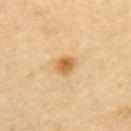follow-up = no biopsy performed (imaged during a skin exam)
patient = female, approximately 45 years of age
body site = the upper back
acquisition = ~15 mm tile from a whole-body skin photo